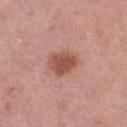Assessment: Part of a total-body skin-imaging series; this lesion was reviewed on a skin check and was not flagged for biopsy. Clinical summary: A female patient approximately 40 years of age. About 3.5 mm across. The lesion is located on the leg. A close-up tile cropped from a whole-body skin photograph, about 15 mm across.